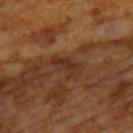Impression: No biopsy was performed on this lesion — it was imaged during a full skin examination and was not determined to be concerning. Acquisition and patient details: The lesion-visualizer software estimated an area of roughly 5 mm² and a symmetry-axis asymmetry near 0.35. The analysis additionally found a border-irregularity rating of about 4/10. A male subject, aged 63 to 67. A 15 mm crop from a total-body photograph taken for skin-cancer surveillance. The lesion's longest dimension is about 3.5 mm. The tile uses cross-polarized illumination.